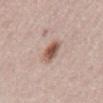The lesion was tiled from a total-body skin photograph and was not biopsied.
The lesion's longest dimension is about 4 mm.
A close-up tile cropped from a whole-body skin photograph, about 15 mm across.
The lesion is on the mid back.
A male subject, in their 50s.